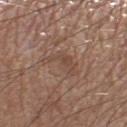The lesion was photographed on a routine skin check and not biopsied; there is no pathology result.
The lesion-visualizer software estimated a lesion area of about 7.5 mm² and two-axis asymmetry of about 0.55. The software also gave a lesion color around L≈47 a*≈18 b*≈25 in CIELAB and a normalized border contrast of about 4.5. It also reported internal color variation of about 2.5 on a 0–10 scale and peripheral color asymmetry of about 1.
This is a white-light tile.
The subject is a male aged 53 to 57.
Cropped from a total-body skin-imaging series; the visible field is about 15 mm.
Located on the right lower leg.